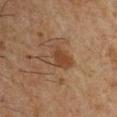Clinical impression:
Part of a total-body skin-imaging series; this lesion was reviewed on a skin check and was not flagged for biopsy.
Context:
Cropped from a whole-body photographic skin survey; the tile spans about 15 mm. From the left upper arm. The subject is a male aged 48 to 52. The lesion's longest dimension is about 4 mm. The tile uses cross-polarized illumination.